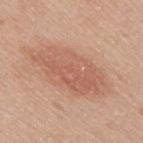This lesion was catalogued during total-body skin photography and was not selected for biopsy. A male patient, aged around 45. A 15 mm close-up extracted from a 3D total-body photography capture. The lesion is on the right upper arm. Captured under white-light illumination. The lesion's longest dimension is about 9.5 mm.